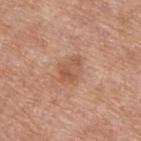notes: catalogued during a skin exam; not biopsied | location: the upper back | imaging modality: total-body-photography crop, ~15 mm field of view | subject: male, aged approximately 55 | automated lesion analysis: an area of roughly 6.5 mm² and a shape-asymmetry score of about 0.25 (0 = symmetric); a mean CIELAB color near L≈55 a*≈21 b*≈32 and a normalized lesion–skin contrast near 5.5; internal color variation of about 4 on a 0–10 scale and a peripheral color-asymmetry measure near 1.5 | diameter: about 3.5 mm | tile lighting: white-light illumination.